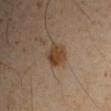Clinical impression:
Part of a total-body skin-imaging series; this lesion was reviewed on a skin check and was not flagged for biopsy.
Context:
A 15 mm close-up tile from a total-body photography series done for melanoma screening. A male patient about 50 years old. From the left upper arm.